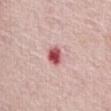Clinical impression:
This lesion was catalogued during total-body skin photography and was not selected for biopsy.
Acquisition and patient details:
From the abdomen. A region of skin cropped from a whole-body photographic capture, roughly 15 mm wide. This is a white-light tile. The lesion-visualizer software estimated internal color variation of about 6 on a 0–10 scale and radial color variation of about 2. And it measured a detector confidence of about 100 out of 100 that the crop contains a lesion. The subject is a female in their mid-60s.